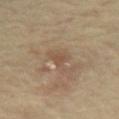Clinical impression:
This lesion was catalogued during total-body skin photography and was not selected for biopsy.
Clinical summary:
The lesion is located on the abdomen. Cropped from a whole-body photographic skin survey; the tile spans about 15 mm. Measured at roughly 2 mm in maximum diameter. The subject is a male aged 68–72. Imaged with cross-polarized lighting.